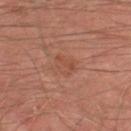The lesion was photographed on a routine skin check and not biopsied; there is no pathology result.
A male subject, aged approximately 65.
A lesion tile, about 15 mm wide, cut from a 3D total-body photograph.
Imaged with cross-polarized lighting.
Longest diameter approximately 2.5 mm.
On the left thigh.